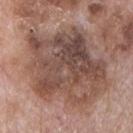subject: male, approximately 70 years of age | automated metrics: an eccentricity of roughly 0.55 and two-axis asymmetry of about 0.25; an average lesion color of about L≈48 a*≈18 b*≈24 (CIELAB) and a lesion-to-skin contrast of about 8.5 (normalized; higher = more distinct); a within-lesion color-variation index near 8.5/10 and peripheral color asymmetry of about 3; an automated nevus-likeness rating near 0 out of 100 and lesion-presence confidence of about 100/100 | acquisition: ~15 mm crop, total-body skin-cancer survey | size: about 10.5 mm | location: the chest.